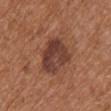No biopsy was performed on this lesion — it was imaged during a full skin examination and was not determined to be concerning.
A male patient aged around 70.
On the upper back.
This is a white-light tile.
A close-up tile cropped from a whole-body skin photograph, about 15 mm across.
Approximately 5 mm at its widest.